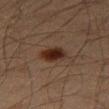notes = total-body-photography surveillance lesion; no biopsy
subject = male, aged 58–62
lighting = cross-polarized illumination
anatomic site = the leg
imaging modality = total-body-photography crop, ~15 mm field of view
diameter = ~3.5 mm (longest diameter)